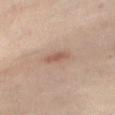Notes:
• notes · catalogued during a skin exam; not biopsied
• image source · ~15 mm tile from a whole-body skin photo
• size · about 3.5 mm
• subject · female, aged around 40
• location · the abdomen
• tile lighting · cross-polarized